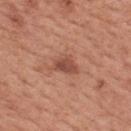Impression: This lesion was catalogued during total-body skin photography and was not selected for biopsy. Background: The lesion is located on the mid back. This image is a 15 mm lesion crop taken from a total-body photograph. Captured under white-light illumination. The patient is a male roughly 55 years of age. The lesion's longest dimension is about 3 mm.